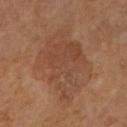notes=imaged on a skin check; not biopsied | image source=total-body-photography crop, ~15 mm field of view | subject=female, about 65 years old | anatomic site=the right forearm.